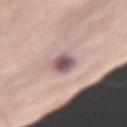Findings:
• workup: imaged on a skin check; not biopsied
• image: total-body-photography crop, ~15 mm field of view
• tile lighting: white-light illumination
• patient: male, approximately 75 years of age
• size: ~3 mm (longest diameter)
• site: the right forearm